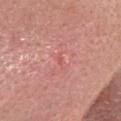A female subject, roughly 65 years of age. Cropped from a whole-body photographic skin survey; the tile spans about 15 mm. Imaged with white-light lighting. Approximately 1.5 mm at its widest. From the head or neck. Histopathologically confirmed as a nodular basal cell carcinoma.